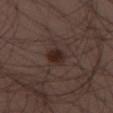Clinical impression: No biopsy was performed on this lesion — it was imaged during a full skin examination and was not determined to be concerning. Context: Captured under white-light illumination. This image is a 15 mm lesion crop taken from a total-body photograph. An algorithmic analysis of the crop reported a lesion area of about 5 mm² and an outline eccentricity of about 0.7 (0 = round, 1 = elongated). And it measured border irregularity of about 1 on a 0–10 scale, internal color variation of about 3 on a 0–10 scale, and radial color variation of about 1. A male patient in their 40s. Measured at roughly 3 mm in maximum diameter. From the left thigh.